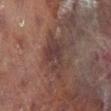notes: catalogued during a skin exam; not biopsied | image: 15 mm crop, total-body photography | size: about 5.5 mm | location: the left leg | patient: female, aged around 80.